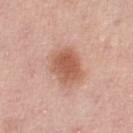Q: Was this lesion biopsied?
A: no biopsy performed (imaged during a skin exam)
Q: How large is the lesion?
A: ~4.5 mm (longest diameter)
Q: What kind of image is this?
A: 15 mm crop, total-body photography
Q: What is the anatomic site?
A: the left thigh
Q: What are the patient's age and sex?
A: female, aged approximately 50
Q: Automated lesion metrics?
A: an automated nevus-likeness rating near 95 out of 100 and a detector confidence of about 100 out of 100 that the crop contains a lesion
Q: How was the tile lit?
A: white-light illumination